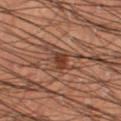Recorded during total-body skin imaging; not selected for excision or biopsy. The lesion is on the right thigh. A 15 mm close-up extracted from a 3D total-body photography capture. A male patient approximately 55 years of age. Longest diameter approximately 2.5 mm. This is a cross-polarized tile.